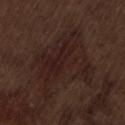Recorded during total-body skin imaging; not selected for excision or biopsy.
A male patient roughly 70 years of age.
This is a white-light tile.
A region of skin cropped from a whole-body photographic capture, roughly 15 mm wide.
Longest diameter approximately 8.5 mm.
Located on the lower back.
Automated tile analysis of the lesion measured an automated nevus-likeness rating near 0 out of 100 and lesion-presence confidence of about 65/100.